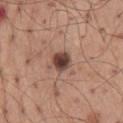No biopsy was performed on this lesion — it was imaged during a full skin examination and was not determined to be concerning.
This is a white-light tile.
From the back.
A lesion tile, about 15 mm wide, cut from a 3D total-body photograph.
A male subject approximately 60 years of age.